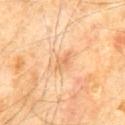Q: Was a biopsy performed?
A: imaged on a skin check; not biopsied
Q: What lighting was used for the tile?
A: cross-polarized illumination
Q: What is the lesion's diameter?
A: ≈4 mm
Q: What kind of image is this?
A: total-body-photography crop, ~15 mm field of view
Q: Patient demographics?
A: male, aged around 60
Q: Lesion location?
A: the front of the torso